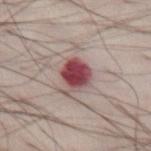illumination: white-light illumination
patient: male, aged 38 to 42
anatomic site: the abdomen
image-analysis metrics: a mean CIELAB color near L≈47 a*≈27 b*≈18, about 18 CIELAB-L* units darker than the surrounding skin, and a lesion-to-skin contrast of about 12.5 (normalized; higher = more distinct); border irregularity of about 3 on a 0–10 scale and a color-variation rating of about 6/10
diameter: ≈4 mm
imaging modality: 15 mm crop, total-body photography